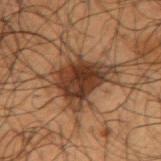| field | value |
|---|---|
| follow-up | total-body-photography surveillance lesion; no biopsy |
| image | ~15 mm tile from a whole-body skin photo |
| site | the right upper arm |
| subject | male, approximately 50 years of age |